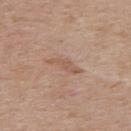Q: Is there a histopathology result?
A: total-body-photography surveillance lesion; no biopsy
Q: How large is the lesion?
A: about 4 mm
Q: Automated lesion metrics?
A: a lesion–skin lightness drop of about 7 and a normalized lesion–skin contrast near 5.5; a nevus-likeness score of about 0/100
Q: Patient demographics?
A: male, roughly 70 years of age
Q: Illumination type?
A: white-light
Q: What kind of image is this?
A: total-body-photography crop, ~15 mm field of view
Q: What is the anatomic site?
A: the upper back